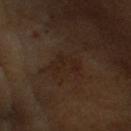Q: Is there a histopathology result?
A: catalogued during a skin exam; not biopsied
Q: Automated lesion metrics?
A: an eccentricity of roughly 0.65 and two-axis asymmetry of about 0.4; a classifier nevus-likeness of about 0/100 and a lesion-detection confidence of about 95/100
Q: Lesion location?
A: the head or neck
Q: What is the lesion's diameter?
A: about 3.5 mm
Q: What are the patient's age and sex?
A: male, aged approximately 65
Q: What lighting was used for the tile?
A: cross-polarized illumination
Q: What is the imaging modality?
A: ~15 mm crop, total-body skin-cancer survey